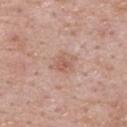Q: Was this lesion biopsied?
A: imaged on a skin check; not biopsied